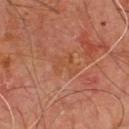Impression:
The lesion was tiled from a total-body skin photograph and was not biopsied.
Acquisition and patient details:
Located on the chest. The subject is a male aged 63 to 67. Cropped from a total-body skin-imaging series; the visible field is about 15 mm.